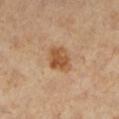Q: Was a biopsy performed?
A: total-body-photography surveillance lesion; no biopsy
Q: Lesion location?
A: the left lower leg
Q: Who is the patient?
A: female, about 45 years old
Q: What is the imaging modality?
A: ~15 mm crop, total-body skin-cancer survey